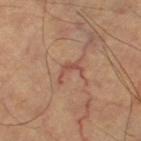Clinical impression:
Captured during whole-body skin photography for melanoma surveillance; the lesion was not biopsied.
Context:
Imaged with cross-polarized lighting. This image is a 15 mm lesion crop taken from a total-body photograph. Located on the right thigh. A subject aged around 65. The recorded lesion diameter is about 3.5 mm.